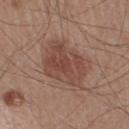Imaged during a routine full-body skin examination; the lesion was not biopsied and no histopathology is available. On the right lower leg. The total-body-photography lesion software estimated a lesion area of about 21 mm², an eccentricity of roughly 0.75, and a symmetry-axis asymmetry near 0.15. And it measured roughly 9 lightness units darker than nearby skin and a lesion-to-skin contrast of about 7 (normalized; higher = more distinct). The software also gave a nevus-likeness score of about 45/100. A male patient in their mid-40s. Captured under white-light illumination. A close-up tile cropped from a whole-body skin photograph, about 15 mm across. About 6.5 mm across.